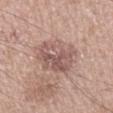follow-up: imaged on a skin check; not biopsied | automated lesion analysis: a lesion area of about 17 mm², a shape eccentricity near 0.6, and a symmetry-axis asymmetry near 0.2; a nevus-likeness score of about 10/100 | patient: male, approximately 50 years of age | anatomic site: the right lower leg | acquisition: 15 mm crop, total-body photography | tile lighting: white-light illumination | lesion diameter: about 5 mm.